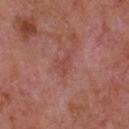{
  "biopsy_status": "not biopsied; imaged during a skin examination",
  "lesion_size": {
    "long_diameter_mm_approx": 3.0
  },
  "image": {
    "source": "total-body photography crop",
    "field_of_view_mm": 15
  },
  "patient": {
    "sex": "male",
    "age_approx": 65
  },
  "lighting": "white-light",
  "site": "front of the torso"
}